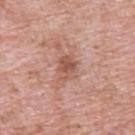No biopsy was performed on this lesion — it was imaged during a full skin examination and was not determined to be concerning. About 3 mm across. The patient is a male aged 48–52. The lesion is on the upper back. Imaged with white-light lighting. A roughly 15 mm field-of-view crop from a total-body skin photograph.